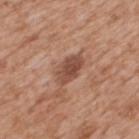<tbp_lesion>
<biopsy_status>not biopsied; imaged during a skin examination</biopsy_status>
<image>
  <source>total-body photography crop</source>
  <field_of_view_mm>15</field_of_view_mm>
</image>
<site>mid back</site>
<lighting>white-light</lighting>
<patient>
  <sex>male</sex>
  <age_approx>65</age_approx>
</patient>
</tbp_lesion>